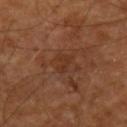Clinical impression: Imaged during a routine full-body skin examination; the lesion was not biopsied and no histopathology is available. Image and clinical context: Automated tile analysis of the lesion measured an area of roughly 3.5 mm² and a shape eccentricity near 0.7. The analysis additionally found a mean CIELAB color near L≈33 a*≈21 b*≈30 and a normalized lesion–skin contrast near 5. And it measured a border-irregularity rating of about 4.5/10 and peripheral color asymmetry of about 0. A 15 mm close-up extracted from a 3D total-body photography capture. The lesion is on the left forearm. About 2.5 mm across. The subject is in their mid- to late 60s.